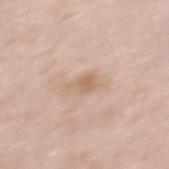Recorded during total-body skin imaging; not selected for excision or biopsy. A 15 mm close-up extracted from a 3D total-body photography capture. On the upper back. A female subject in their mid- to late 60s. Captured under white-light illumination.